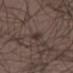Captured during whole-body skin photography for melanoma surveillance; the lesion was not biopsied.
An algorithmic analysis of the crop reported a footprint of about 3 mm² and a shape-asymmetry score of about 0.25 (0 = symmetric). And it measured a lesion color around L≈33 a*≈12 b*≈18 in CIELAB, roughly 7 lightness units darker than nearby skin, and a lesion-to-skin contrast of about 7 (normalized; higher = more distinct).
A male subject, aged around 40.
The tile uses white-light illumination.
Located on the right lower leg.
Approximately 3 mm at its widest.
A close-up tile cropped from a whole-body skin photograph, about 15 mm across.